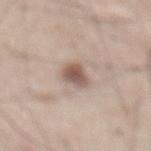| key | value |
|---|---|
| follow-up | imaged on a skin check; not biopsied |
| image | ~15 mm tile from a whole-body skin photo |
| subject | male, aged approximately 65 |
| site | the abdomen |